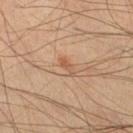Assessment: Recorded during total-body skin imaging; not selected for excision or biopsy. Background: The subject is a male aged approximately 45. Measured at roughly 2.5 mm in maximum diameter. The total-body-photography lesion software estimated an area of roughly 2 mm² and a shape eccentricity near 0.9. The analysis additionally found a border-irregularity rating of about 5.5/10, a color-variation rating of about 0/10, and radial color variation of about 0. Cropped from a total-body skin-imaging series; the visible field is about 15 mm. Captured under cross-polarized illumination. Located on the right lower leg.